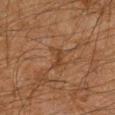{"lighting": "cross-polarized", "patient": {"sex": "male", "age_approx": 50}, "image": {"source": "total-body photography crop", "field_of_view_mm": 15}, "site": "arm", "lesion_size": {"long_diameter_mm_approx": 2.5}}